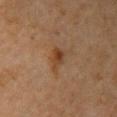| feature | finding |
|---|---|
| site | the arm |
| subject | female, about 70 years old |
| image source | 15 mm crop, total-body photography |